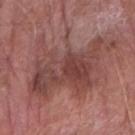{"biopsy_status": "not biopsied; imaged during a skin examination", "image": {"source": "total-body photography crop", "field_of_view_mm": 15}, "lesion_size": {"long_diameter_mm_approx": 11.0}, "site": "right forearm", "patient": {"sex": "male", "age_approx": 80}, "lighting": "white-light"}